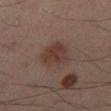notes — imaged on a skin check; not biopsied | patient — male, in their 40s | acquisition — total-body-photography crop, ~15 mm field of view | location — the right lower leg.